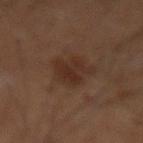Captured during whole-body skin photography for melanoma surveillance; the lesion was not biopsied.
On the mid back.
The patient is a male roughly 60 years of age.
This is a cross-polarized tile.
Automated tile analysis of the lesion measured a classifier nevus-likeness of about 35/100 and a lesion-detection confidence of about 100/100.
A roughly 15 mm field-of-view crop from a total-body skin photograph.